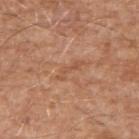Q: What is the anatomic site?
A: the right upper arm
Q: Who is the patient?
A: male, roughly 60 years of age
Q: What kind of image is this?
A: 15 mm crop, total-body photography